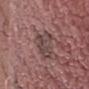Assessment:
Part of a total-body skin-imaging series; this lesion was reviewed on a skin check and was not flagged for biopsy.
Image and clinical context:
Automated tile analysis of the lesion measured a footprint of about 7.5 mm², an eccentricity of roughly 0.35, and a symmetry-axis asymmetry near 0.55. The lesion is located on the right thigh. A male patient aged around 45. A 15 mm crop from a total-body photograph taken for skin-cancer surveillance. The recorded lesion diameter is about 3.5 mm. Imaged with white-light lighting.